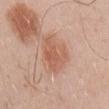Case summary:
– follow-up: imaged on a skin check; not biopsied
– image source: total-body-photography crop, ~15 mm field of view
– subject: male, approximately 30 years of age
– anatomic site: the right upper arm